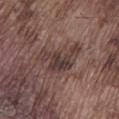<tbp_lesion>
  <biopsy_status>not biopsied; imaged during a skin examination</biopsy_status>
  <site>leg</site>
  <image>
    <source>total-body photography crop</source>
    <field_of_view_mm>15</field_of_view_mm>
  </image>
  <patient>
    <sex>male</sex>
    <age_approx>75</age_approx>
  </patient>
</tbp_lesion>